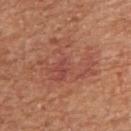* follow-up · catalogued during a skin exam; not biopsied
* lesion diameter · ≈6.5 mm
* image source · ~15 mm tile from a whole-body skin photo
* automated metrics · a lesion area of about 22 mm², an outline eccentricity of about 0.55 (0 = round, 1 = elongated), and two-axis asymmetry of about 0.6; a lesion color around L≈49 a*≈26 b*≈29 in CIELAB, a lesion–skin lightness drop of about 6, and a normalized lesion–skin contrast near 5; a border-irregularity index near 9.5/10, a within-lesion color-variation index near 4.5/10, and peripheral color asymmetry of about 1.5
* patient · male, aged approximately 65
* illumination · white-light
* location · the right upper arm